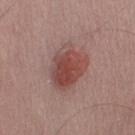Impression: The lesion was tiled from a total-body skin photograph and was not biopsied. Acquisition and patient details: A male patient, approximately 65 years of age. On the right upper arm. Captured under white-light illumination. The total-body-photography lesion software estimated an area of roughly 17 mm², an eccentricity of roughly 0.7, and two-axis asymmetry of about 0.25. It also reported a border-irregularity index near 2.5/10 and a within-lesion color-variation index near 6/10. A 15 mm crop from a total-body photograph taken for skin-cancer surveillance. Approximately 5.5 mm at its widest.